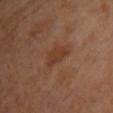No biopsy was performed on this lesion — it was imaged during a full skin examination and was not determined to be concerning. The lesion is located on the chest. Measured at roughly 3.5 mm in maximum diameter. The subject is a female roughly 65 years of age. Cropped from a whole-body photographic skin survey; the tile spans about 15 mm. This is a cross-polarized tile.